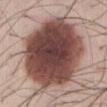This lesion was catalogued during total-body skin photography and was not selected for biopsy. A region of skin cropped from a whole-body photographic capture, roughly 15 mm wide. A male subject, about 40 years old. Imaged with white-light lighting. Approximately 9.5 mm at its widest. From the abdomen.